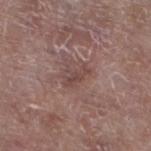This lesion was catalogued during total-body skin photography and was not selected for biopsy.
A male subject approximately 80 years of age.
The lesion is located on the leg.
A lesion tile, about 15 mm wide, cut from a 3D total-body photograph.